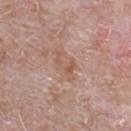The lesion was photographed on a routine skin check and not biopsied; there is no pathology result.